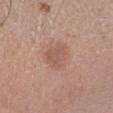No biopsy was performed on this lesion — it was imaged during a full skin examination and was not determined to be concerning.
A 15 mm crop from a total-body photograph taken for skin-cancer surveillance.
Approximately 3.5 mm at its widest.
Imaged with white-light lighting.
A male patient about 55 years old.
Located on the right lower leg.